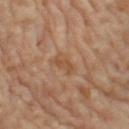| feature | finding |
|---|---|
| biopsy status | total-body-photography surveillance lesion; no biopsy |
| anatomic site | the left thigh |
| patient | female, aged 68 to 72 |
| tile lighting | cross-polarized illumination |
| imaging modality | total-body-photography crop, ~15 mm field of view |
| automated metrics | a lesion color around L≈52 a*≈20 b*≈34 in CIELAB, about 6 CIELAB-L* units darker than the surrounding skin, and a normalized border contrast of about 5.5; border irregularity of about 3.5 on a 0–10 scale, a within-lesion color-variation index near 2/10, and radial color variation of about 0.5 |